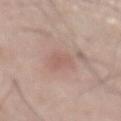{"biopsy_status": "not biopsied; imaged during a skin examination", "lesion_size": {"long_diameter_mm_approx": 3.0}, "patient": {"sex": "male", "age_approx": 75}, "site": "abdomen", "image": {"source": "total-body photography crop", "field_of_view_mm": 15}, "lighting": "white-light"}